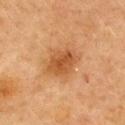biopsy status: no biopsy performed (imaged during a skin exam) | patient: female, in their 60s | lesion size: ~4 mm (longest diameter) | image source: ~15 mm tile from a whole-body skin photo | tile lighting: cross-polarized | location: the upper back.